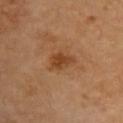| feature | finding |
|---|---|
| patient | female, aged approximately 70 |
| location | the back |
| automated lesion analysis | a mean CIELAB color near L≈41 a*≈22 b*≈35, a lesion–skin lightness drop of about 9, and a normalized border contrast of about 8; border irregularity of about 3 on a 0–10 scale, internal color variation of about 2.5 on a 0–10 scale, and peripheral color asymmetry of about 1; a classifier nevus-likeness of about 55/100 and a detector confidence of about 100 out of 100 that the crop contains a lesion |
| diameter | about 3 mm |
| imaging modality | total-body-photography crop, ~15 mm field of view |